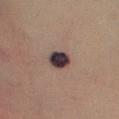Recorded during total-body skin imaging; not selected for excision or biopsy. A close-up tile cropped from a whole-body skin photograph, about 15 mm across. The tile uses cross-polarized illumination. A male subject in their mid-40s. The lesion is on the leg. The recorded lesion diameter is about 2.5 mm.